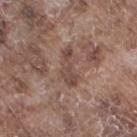<lesion>
  <biopsy_status>not biopsied; imaged during a skin examination</biopsy_status>
  <automated_metrics>
    <area_mm2_approx>5.5</area_mm2_approx>
  </automated_metrics>
  <image>
    <source>total-body photography crop</source>
    <field_of_view_mm>15</field_of_view_mm>
  </image>
  <patient>
    <sex>male</sex>
    <age_approx>75</age_approx>
  </patient>
  <site>right thigh</site>
</lesion>